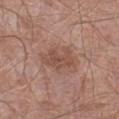The lesion was photographed on a routine skin check and not biopsied; there is no pathology result.